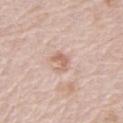acquisition=total-body-photography crop, ~15 mm field of view; patient=male, aged 78 to 82; site=the chest.